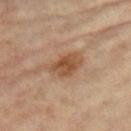This lesion was catalogued during total-body skin photography and was not selected for biopsy.
On the left thigh.
A female subject, in their mid-70s.
A close-up tile cropped from a whole-body skin photograph, about 15 mm across.